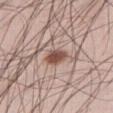Recorded during total-body skin imaging; not selected for excision or biopsy. Longest diameter approximately 3 mm. Located on the right thigh. The tile uses white-light illumination. A male patient aged 33–37. This image is a 15 mm lesion crop taken from a total-body photograph.